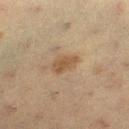TBP lesion metrics: an eccentricity of roughly 0.8 and a symmetry-axis asymmetry near 0.25; a lesion color around L≈43 a*≈14 b*≈29 in CIELAB, a lesion–skin lightness drop of about 8, and a lesion-to-skin contrast of about 7 (normalized; higher = more distinct) | lighting: cross-polarized | image: 15 mm crop, total-body photography | body site: the right lower leg | lesion diameter: ≈3.5 mm | subject: female, about 40 years old.